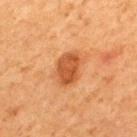The lesion was tiled from a total-body skin photograph and was not biopsied. This is a cross-polarized tile. A close-up tile cropped from a whole-body skin photograph, about 15 mm across. From the upper back. The total-body-photography lesion software estimated a mean CIELAB color near L≈47 a*≈27 b*≈38 and a lesion–skin lightness drop of about 11. A male patient aged 63 to 67. About 4 mm across.